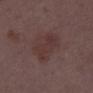The lesion was tiled from a total-body skin photograph and was not biopsied.